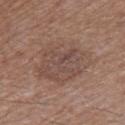workup = imaged on a skin check; not biopsied
subject = male, aged approximately 65
imaging modality = ~15 mm crop, total-body skin-cancer survey
lighting = white-light
site = the right thigh